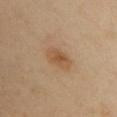Impression: The lesion was photographed on a routine skin check and not biopsied; there is no pathology result. Context: The patient is a female approximately 40 years of age. The lesion is on the chest. This is a cross-polarized tile. Longest diameter approximately 3.5 mm. Cropped from a whole-body photographic skin survey; the tile spans about 15 mm.